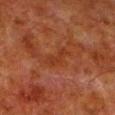Q: Was a biopsy performed?
A: total-body-photography surveillance lesion; no biopsy
Q: What kind of image is this?
A: ~15 mm tile from a whole-body skin photo
Q: Lesion location?
A: the left lower leg
Q: What are the patient's age and sex?
A: male, aged 78 to 82